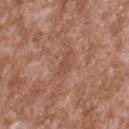• workup: imaged on a skin check; not biopsied
• image source: 15 mm crop, total-body photography
• illumination: white-light
• size: ~3 mm (longest diameter)
• subject: male, aged approximately 45
• anatomic site: the upper back